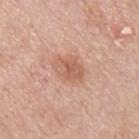biopsy status = total-body-photography surveillance lesion; no biopsy | patient = male, in their mid-50s | image = total-body-photography crop, ~15 mm field of view | body site = the right upper arm | image-analysis metrics = a mean CIELAB color near L≈60 a*≈23 b*≈31 and about 9 CIELAB-L* units darker than the surrounding skin; a border-irregularity index near 1.5/10, a within-lesion color-variation index near 4/10, and a peripheral color-asymmetry measure near 1.5.